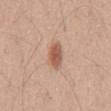The total-body-photography lesion software estimated a footprint of about 5.5 mm², an outline eccentricity of about 0.85 (0 = round, 1 = elongated), and a shape-asymmetry score of about 0.2 (0 = symmetric). And it measured a mean CIELAB color near L≈57 a*≈22 b*≈31, about 12 CIELAB-L* units darker than the surrounding skin, and a lesion-to-skin contrast of about 8.5 (normalized; higher = more distinct). It also reported border irregularity of about 2 on a 0–10 scale, a color-variation rating of about 3/10, and peripheral color asymmetry of about 1. And it measured a classifier nevus-likeness of about 95/100 and a detector confidence of about 100 out of 100 that the crop contains a lesion.
This is a white-light tile.
Approximately 4 mm at its widest.
Located on the abdomen.
A male subject, approximately 55 years of age.
A close-up tile cropped from a whole-body skin photograph, about 15 mm across.